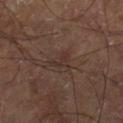follow-up: total-body-photography surveillance lesion; no biopsy
lesion diameter: ~2.5 mm (longest diameter)
tile lighting: cross-polarized
TBP lesion metrics: a lesion color around L≈32 a*≈16 b*≈20 in CIELAB and a normalized lesion–skin contrast near 6; a classifier nevus-likeness of about 0/100 and a detector confidence of about 95 out of 100 that the crop contains a lesion
image source: 15 mm crop, total-body photography
site: the right thigh